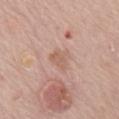This is a white-light tile. The lesion is on the mid back. The lesion's longest dimension is about 2.5 mm. Automated tile analysis of the lesion measured a shape eccentricity near 0.75 and a symmetry-axis asymmetry near 0.3. The analysis additionally found a mean CIELAB color near L≈59 a*≈20 b*≈28, roughly 7 lightness units darker than nearby skin, and a lesion-to-skin contrast of about 5 (normalized; higher = more distinct). And it measured a within-lesion color-variation index near 1.5/10 and radial color variation of about 0.5. A 15 mm close-up extracted from a 3D total-body photography capture. The patient is a female roughly 85 years of age or older.